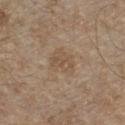Q: Is there a histopathology result?
A: total-body-photography surveillance lesion; no biopsy
Q: Lesion location?
A: the chest
Q: Lesion size?
A: about 3.5 mm
Q: Patient demographics?
A: male, approximately 70 years of age
Q: What did automated image analysis measure?
A: a lesion area of about 8 mm², a shape eccentricity near 0.6, and two-axis asymmetry of about 0.25; an automated nevus-likeness rating near 0 out of 100 and a lesion-detection confidence of about 100/100
Q: What kind of image is this?
A: ~15 mm tile from a whole-body skin photo